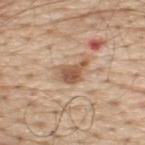<record>
<biopsy_status>not biopsied; imaged during a skin examination</biopsy_status>
<automated_metrics>
  <vs_skin_darker_L>12.0</vs_skin_darker_L>
</automated_metrics>
<lighting>white-light</lighting>
<patient>
  <sex>male</sex>
  <age_approx>70</age_approx>
</patient>
<lesion_size>
  <long_diameter_mm_approx>4.0</long_diameter_mm_approx>
</lesion_size>
<image>
  <source>total-body photography crop</source>
  <field_of_view_mm>15</field_of_view_mm>
</image>
<site>upper back</site>
</record>